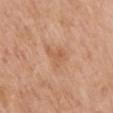{
  "biopsy_status": "not biopsied; imaged during a skin examination",
  "lesion_size": {
    "long_diameter_mm_approx": 3.5
  },
  "image": {
    "source": "total-body photography crop",
    "field_of_view_mm": 15
  },
  "lighting": "white-light",
  "site": "mid back",
  "patient": {
    "sex": "female",
    "age_approx": 65
  }
}